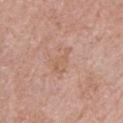Assessment: Part of a total-body skin-imaging series; this lesion was reviewed on a skin check and was not flagged for biopsy. Clinical summary: Located on the left upper arm. Captured under white-light illumination. The lesion-visualizer software estimated a classifier nevus-likeness of about 0/100 and a lesion-detection confidence of about 100/100. The recorded lesion diameter is about 3.5 mm. A male subject approximately 70 years of age. Cropped from a whole-body photographic skin survey; the tile spans about 15 mm.